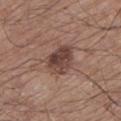Captured during whole-body skin photography for melanoma surveillance; the lesion was not biopsied. Imaged with white-light lighting. A close-up tile cropped from a whole-body skin photograph, about 15 mm across. Longest diameter approximately 4.5 mm. The patient is a male roughly 65 years of age. An algorithmic analysis of the crop reported border irregularity of about 2 on a 0–10 scale, internal color variation of about 5.5 on a 0–10 scale, and peripheral color asymmetry of about 2. And it measured a nevus-likeness score of about 65/100 and a detector confidence of about 100 out of 100 that the crop contains a lesion. The lesion is located on the left lower leg.